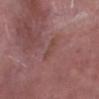notes: total-body-photography surveillance lesion; no biopsy | patient: male, aged approximately 40 | lighting: white-light | anatomic site: the left lower leg | lesion diameter: ≈3 mm | image source: ~15 mm tile from a whole-body skin photo.